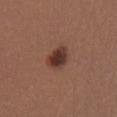biopsy status — total-body-photography surveillance lesion; no biopsy